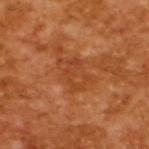Automated tile analysis of the lesion measured a symmetry-axis asymmetry near 0.6. It also reported a mean CIELAB color near L≈43 a*≈29 b*≈39, roughly 6 lightness units darker than nearby skin, and a normalized border contrast of about 5. The software also gave a border-irregularity index near 9.5/10, a color-variation rating of about 0/10, and radial color variation of about 0. The lesion's longest dimension is about 3.5 mm. A male subject roughly 65 years of age. This is a cross-polarized tile. A lesion tile, about 15 mm wide, cut from a 3D total-body photograph.